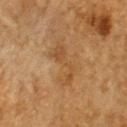Acquisition and patient details:
A 15 mm close-up extracted from a 3D total-body photography capture. The lesion's longest dimension is about 5 mm. A male subject aged around 85 or older. The total-body-photography lesion software estimated a lesion area of about 8 mm², a shape eccentricity near 0.9, and a symmetry-axis asymmetry near 0.5. The software also gave an automated nevus-likeness rating near 0 out of 100 and a lesion-detection confidence of about 100/100. From the upper back.